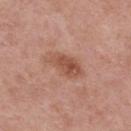Recorded during total-body skin imaging; not selected for excision or biopsy.
An algorithmic analysis of the crop reported a footprint of about 9 mm², an eccentricity of roughly 0.85, and a shape-asymmetry score of about 0.25 (0 = symmetric). The software also gave a lesion color around L≈52 a*≈23 b*≈30 in CIELAB and a normalized lesion–skin contrast near 7.
Cropped from a whole-body photographic skin survey; the tile spans about 15 mm.
The patient is a male in their mid- to late 60s.
The recorded lesion diameter is about 5 mm.
The lesion is located on the upper back.
This is a white-light tile.